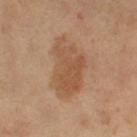Captured during whole-body skin photography for melanoma surveillance; the lesion was not biopsied. Cropped from a whole-body photographic skin survey; the tile spans about 15 mm. On the left thigh. Longest diameter approximately 6.5 mm. An algorithmic analysis of the crop reported a border-irregularity index near 4.5/10, a within-lesion color-variation index near 3.5/10, and radial color variation of about 1. The software also gave a classifier nevus-likeness of about 30/100. Imaged with cross-polarized lighting. The patient is a female aged 53–57.